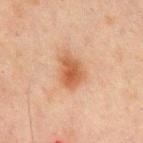Clinical impression: This lesion was catalogued during total-body skin photography and was not selected for biopsy. Background: A roughly 15 mm field-of-view crop from a total-body skin photograph. The subject is a male aged approximately 70. About 4 mm across. The lesion-visualizer software estimated an area of roughly 8 mm², a shape eccentricity near 0.7, and a symmetry-axis asymmetry near 0.25. On the back. Imaged with cross-polarized lighting.